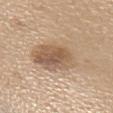biopsy status=total-body-photography surveillance lesion; no biopsy | automated metrics=a classifier nevus-likeness of about 60/100 and a lesion-detection confidence of about 100/100 | tile lighting=white-light | location=the upper back | imaging modality=15 mm crop, total-body photography | patient=female, aged 58 to 62.